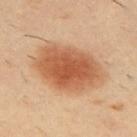Case summary:
• imaging modality: ~15 mm tile from a whole-body skin photo
• subject: male, aged around 40
• body site: the back
• size: ~8 mm (longest diameter)
• illumination: cross-polarized illumination